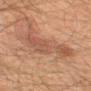* workup: catalogued during a skin exam; not biopsied
* tile lighting: cross-polarized
* location: the mid back
* acquisition: ~15 mm tile from a whole-body skin photo
* subject: male, aged 58 to 62